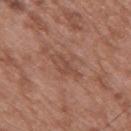Notes:
• workup · imaged on a skin check; not biopsied
• lesion diameter · about 4 mm
• patient · male, roughly 50 years of age
• imaging modality · 15 mm crop, total-body photography
• anatomic site · the mid back
• lighting · white-light illumination